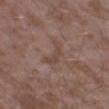Impression:
Captured during whole-body skin photography for melanoma surveillance; the lesion was not biopsied.
Background:
A male patient roughly 45 years of age. This is a white-light tile. The total-body-photography lesion software estimated a footprint of about 5 mm², an eccentricity of roughly 0.85, and a symmetry-axis asymmetry near 0.55. The analysis additionally found a mean CIELAB color near L≈45 a*≈17 b*≈23, a lesion–skin lightness drop of about 6, and a normalized lesion–skin contrast near 5. It also reported a border-irregularity rating of about 6/10, a color-variation rating of about 1/10, and a peripheral color-asymmetry measure near 0. It also reported a nevus-likeness score of about 0/100 and a lesion-detection confidence of about 100/100. A 15 mm crop from a total-body photograph taken for skin-cancer surveillance. The lesion is on the leg.